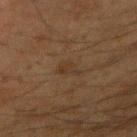<lesion>
<biopsy_status>not biopsied; imaged during a skin examination</biopsy_status>
<automated_metrics>
  <border_irregularity_0_10>4.5</border_irregularity_0_10>
  <color_variation_0_10>1.5</color_variation_0_10>
  <peripheral_color_asymmetry>0.5</peripheral_color_asymmetry>
  <nevus_likeness_0_100>0</nevus_likeness_0_100>
  <lesion_detection_confidence_0_100>100</lesion_detection_confidence_0_100>
</automated_metrics>
<site>right upper arm</site>
<patient>
  <sex>male</sex>
  <age_approx>35</age_approx>
</patient>
<lesion_size>
  <long_diameter_mm_approx>2.5</long_diameter_mm_approx>
</lesion_size>
<image>
  <source>total-body photography crop</source>
  <field_of_view_mm>15</field_of_view_mm>
</image>
</lesion>